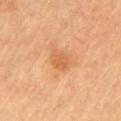Clinical impression:
The lesion was photographed on a routine skin check and not biopsied; there is no pathology result.
Acquisition and patient details:
The tile uses cross-polarized illumination. Cropped from a total-body skin-imaging series; the visible field is about 15 mm. A male patient, approximately 85 years of age or older. From the chest. The total-body-photography lesion software estimated two-axis asymmetry of about 0.2. The analysis additionally found a nevus-likeness score of about 5/100.